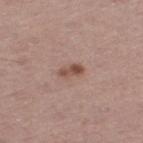Notes:
• biopsy status · total-body-photography surveillance lesion; no biopsy
• site · the left thigh
• imaging modality · ~15 mm crop, total-body skin-cancer survey
• patient · male, approximately 55 years of age